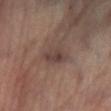follow-up: total-body-photography surveillance lesion; no biopsy
size: ≈3.5 mm
tile lighting: cross-polarized
anatomic site: the left lower leg
acquisition: ~15 mm crop, total-body skin-cancer survey